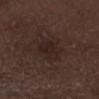Impression:
The lesion was photographed on a routine skin check and not biopsied; there is no pathology result.
Acquisition and patient details:
Imaged with white-light lighting. On the leg. The subject is a male aged 68 to 72. The recorded lesion diameter is about 3 mm. A lesion tile, about 15 mm wide, cut from a 3D total-body photograph.